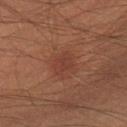Clinical summary: A 15 mm crop from a total-body photograph taken for skin-cancer surveillance. Approximately 2.5 mm at its widest. Imaged with cross-polarized lighting. Automated image analysis of the tile measured a classifier nevus-likeness of about 80/100 and a lesion-detection confidence of about 100/100. The patient is a male aged 38–42. The lesion is on the right lower leg.